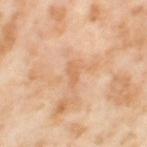No biopsy was performed on this lesion — it was imaged during a full skin examination and was not determined to be concerning.